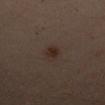About 2.5 mm across.
Imaged with cross-polarized lighting.
A female patient roughly 40 years of age.
From the right upper arm.
Cropped from a total-body skin-imaging series; the visible field is about 15 mm.